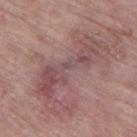Clinical impression:
This lesion was catalogued during total-body skin photography and was not selected for biopsy.
Clinical summary:
Measured at roughly 10 mm in maximum diameter. The subject is a female in their mid-80s or older. The lesion is on the left thigh. Cropped from a total-body skin-imaging series; the visible field is about 15 mm.